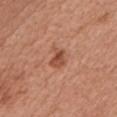The lesion is on the front of the torso. A female patient, in their mid- to late 60s. The lesion-visualizer software estimated an area of roughly 4.5 mm², a shape eccentricity near 0.65, and a shape-asymmetry score of about 0.4 (0 = symmetric). The software also gave an average lesion color of about L≈50 a*≈26 b*≈32 (CIELAB), a lesion–skin lightness drop of about 10, and a lesion-to-skin contrast of about 7.5 (normalized; higher = more distinct). Measured at roughly 2.5 mm in maximum diameter. A 15 mm close-up extracted from a 3D total-body photography capture.